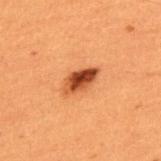The total-body-photography lesion software estimated a footprint of about 6.5 mm², an outline eccentricity of about 0.85 (0 = round, 1 = elongated), and a symmetry-axis asymmetry near 0.2. The analysis additionally found a lesion color around L≈40 a*≈26 b*≈35 in CIELAB, a lesion–skin lightness drop of about 15, and a normalized border contrast of about 11.5. And it measured a border-irregularity rating of about 2/10, a within-lesion color-variation index near 7/10, and a peripheral color-asymmetry measure near 3. A male patient aged around 50. A region of skin cropped from a whole-body photographic capture, roughly 15 mm wide. Located on the upper back.